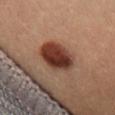{"biopsy_status": "not biopsied; imaged during a skin examination", "patient": {"sex": "female", "age_approx": 25}, "image": {"source": "total-body photography crop", "field_of_view_mm": 15}, "site": "left thigh"}